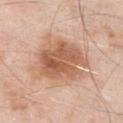Part of a total-body skin-imaging series; this lesion was reviewed on a skin check and was not flagged for biopsy. From the chest. The patient is a male approximately 55 years of age. Imaged with white-light lighting. A lesion tile, about 15 mm wide, cut from a 3D total-body photograph.